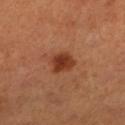Assessment:
Part of a total-body skin-imaging series; this lesion was reviewed on a skin check and was not flagged for biopsy.
Context:
Captured under cross-polarized illumination. Measured at roughly 3 mm in maximum diameter. On the leg. A male patient approximately 50 years of age. A region of skin cropped from a whole-body photographic capture, roughly 15 mm wide.